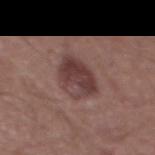This lesion was catalogued during total-body skin photography and was not selected for biopsy.
Captured under white-light illumination.
The total-body-photography lesion software estimated a lesion area of about 13 mm² and an eccentricity of roughly 0.6. It also reported a color-variation rating of about 6.5/10 and a peripheral color-asymmetry measure near 2.5.
A region of skin cropped from a whole-body photographic capture, roughly 15 mm wide.
Located on the abdomen.
Measured at roughly 4.5 mm in maximum diameter.
A male subject in their mid-50s.